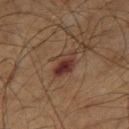Assessment: The lesion was tiled from a total-body skin photograph and was not biopsied. Acquisition and patient details: The patient is a male aged around 60. The recorded lesion diameter is about 3.5 mm. A 15 mm crop from a total-body photograph taken for skin-cancer surveillance. The lesion is located on the left thigh. This is a cross-polarized tile.